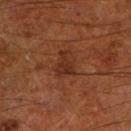No biopsy was performed on this lesion — it was imaged during a full skin examination and was not determined to be concerning. A male subject aged around 65. Cropped from a whole-body photographic skin survey; the tile spans about 15 mm. The tile uses cross-polarized illumination. From the left lower leg. Longest diameter approximately 3 mm. Automated tile analysis of the lesion measured an area of roughly 4.5 mm², an eccentricity of roughly 0.75, and a symmetry-axis asymmetry near 0.6. It also reported a mean CIELAB color near L≈29 a*≈23 b*≈29, about 7 CIELAB-L* units darker than the surrounding skin, and a normalized lesion–skin contrast near 6.5. It also reported border irregularity of about 6.5 on a 0–10 scale and radial color variation of about 0.5.